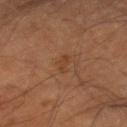| field | value |
|---|---|
| notes | catalogued during a skin exam; not biopsied |
| location | the leg |
| tile lighting | cross-polarized |
| lesion size | ~2.5 mm (longest diameter) |
| subject | male, aged around 65 |
| image source | 15 mm crop, total-body photography |